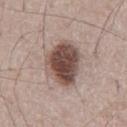<record>
<biopsy_status>not biopsied; imaged during a skin examination</biopsy_status>
<image>
  <source>total-body photography crop</source>
  <field_of_view_mm>15</field_of_view_mm>
</image>
<patient>
  <sex>male</sex>
  <age_approx>55</age_approx>
</patient>
<lesion_size>
  <long_diameter_mm_approx>5.5</long_diameter_mm_approx>
</lesion_size>
<automated_metrics>
  <eccentricity>0.7</eccentricity>
  <shape_asymmetry>0.2</shape_asymmetry>
  <border_irregularity_0_10>2.0</border_irregularity_0_10>
  <color_variation_0_10>6.0</color_variation_0_10>
  <peripheral_color_asymmetry>2.0</peripheral_color_asymmetry>
</automated_metrics>
</record>